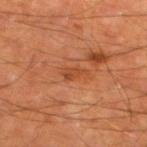Findings:
• biopsy status: no biopsy performed (imaged during a skin exam)
• subject: male, approximately 65 years of age
• imaging modality: 15 mm crop, total-body photography
• anatomic site: the left lower leg